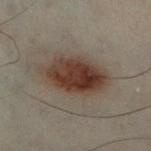notes — catalogued during a skin exam; not biopsied
site — the left leg
TBP lesion metrics — an average lesion color of about L≈30 a*≈12 b*≈20 (CIELAB), roughly 11 lightness units darker than nearby skin, and a normalized lesion–skin contrast near 11.5; a border-irregularity index near 2/10, internal color variation of about 6 on a 0–10 scale, and a peripheral color-asymmetry measure near 2; a lesion-detection confidence of about 100/100
diameter — ≈8 mm
imaging modality — ~15 mm tile from a whole-body skin photo
patient — male, aged 48–52